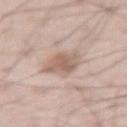Clinical impression: No biopsy was performed on this lesion — it was imaged during a full skin examination and was not determined to be concerning. Acquisition and patient details: A 15 mm close-up extracted from a 3D total-body photography capture. From the left thigh. A male patient, about 55 years old.